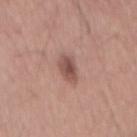No biopsy was performed on this lesion — it was imaged during a full skin examination and was not determined to be concerning. A 15 mm crop from a total-body photograph taken for skin-cancer surveillance. Approximately 4.5 mm at its widest. A male subject, aged around 55. This is a white-light tile.